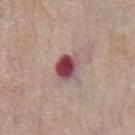Q: Is there a histopathology result?
A: imaged on a skin check; not biopsied
Q: What lighting was used for the tile?
A: white-light illumination
Q: Patient demographics?
A: male, aged approximately 65
Q: Where on the body is the lesion?
A: the chest
Q: Lesion size?
A: ≈3.5 mm
Q: What is the imaging modality?
A: total-body-photography crop, ~15 mm field of view